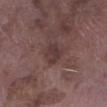<case>
<patient>
  <sex>male</sex>
  <age_approx>75</age_approx>
</patient>
<image>
  <source>total-body photography crop</source>
  <field_of_view_mm>15</field_of_view_mm>
</image>
<site>left lower leg</site>
</case>